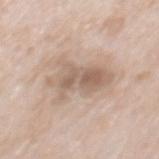Case summary:
* workup: imaged on a skin check; not biopsied
* image source: total-body-photography crop, ~15 mm field of view
* subject: male, roughly 65 years of age
* size: about 6 mm
* site: the mid back
* illumination: white-light